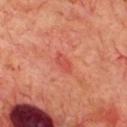biopsy status: catalogued during a skin exam; not biopsied
acquisition: ~15 mm tile from a whole-body skin photo
patient: male, about 70 years old
automated lesion analysis: a footprint of about 3 mm², a shape eccentricity near 0.9, and two-axis asymmetry of about 0.3; a border-irregularity index near 2.5/10, a color-variation rating of about 0.5/10, and peripheral color asymmetry of about 0; a classifier nevus-likeness of about 0/100 and lesion-presence confidence of about 100/100
body site: the chest
lesion diameter: ≈2.5 mm